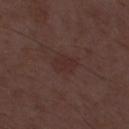Clinical impression:
The lesion was photographed on a routine skin check and not biopsied; there is no pathology result.
Acquisition and patient details:
A male subject, about 50 years old. Cropped from a total-body skin-imaging series; the visible field is about 15 mm.